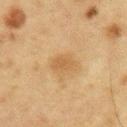Recorded during total-body skin imaging; not selected for excision or biopsy.
The lesion is on the chest.
Imaged with cross-polarized lighting.
About 3.5 mm across.
A male subject in their mid-60s.
A 15 mm close-up tile from a total-body photography series done for melanoma screening.